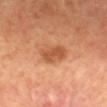Acquisition and patient details:
Captured under cross-polarized illumination. Cropped from a total-body skin-imaging series; the visible field is about 15 mm. Measured at roughly 4 mm in maximum diameter. The lesion is on the mid back. An algorithmic analysis of the crop reported a lesion area of about 7 mm², an eccentricity of roughly 0.8, and a symmetry-axis asymmetry near 0.25. The analysis additionally found a lesion color around L≈56 a*≈28 b*≈39 in CIELAB, a lesion–skin lightness drop of about 11, and a lesion-to-skin contrast of about 7 (normalized; higher = more distinct). The software also gave a border-irregularity index near 2.5/10 and a within-lesion color-variation index near 2/10. It also reported an automated nevus-likeness rating near 90 out of 100 and a detector confidence of about 100 out of 100 that the crop contains a lesion. A female patient approximately 60 years of age.